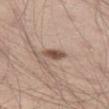Q: Is there a histopathology result?
A: total-body-photography surveillance lesion; no biopsy
Q: How was this image acquired?
A: ~15 mm tile from a whole-body skin photo
Q: How large is the lesion?
A: ~3 mm (longest diameter)
Q: What did automated image analysis measure?
A: a mean CIELAB color near L≈51 a*≈18 b*≈25 and a normalized border contrast of about 9; a nevus-likeness score of about 90/100 and a lesion-detection confidence of about 100/100
Q: Where on the body is the lesion?
A: the left thigh
Q: What are the patient's age and sex?
A: male, approximately 35 years of age
Q: Illumination type?
A: white-light